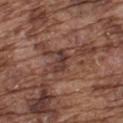No biopsy was performed on this lesion — it was imaged during a full skin examination and was not determined to be concerning.
The lesion's longest dimension is about 3 mm.
A male subject, about 75 years old.
The tile uses white-light illumination.
On the upper back.
A 15 mm close-up extracted from a 3D total-body photography capture.
Automated tile analysis of the lesion measured a mean CIELAB color near L≈36 a*≈20 b*≈22 and a normalized lesion–skin contrast near 8.